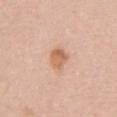Assessment:
Captured during whole-body skin photography for melanoma surveillance; the lesion was not biopsied.
Clinical summary:
A 15 mm close-up tile from a total-body photography series done for melanoma screening. An algorithmic analysis of the crop reported an automated nevus-likeness rating near 90 out of 100. A female subject about 40 years old. The lesion is located on the front of the torso. This is a white-light tile. Measured at roughly 3 mm in maximum diameter.